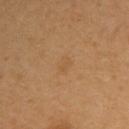biopsy status — catalogued during a skin exam; not biopsied.